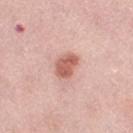<case>
<biopsy_status>not biopsied; imaged during a skin examination</biopsy_status>
<lighting>white-light</lighting>
<patient>
  <sex>female</sex>
  <age_approx>65</age_approx>
</patient>
<image>
  <source>total-body photography crop</source>
  <field_of_view_mm>15</field_of_view_mm>
</image>
<automated_metrics>
  <cielab_L>61</cielab_L>
  <cielab_a>26</cielab_a>
  <cielab_b>28</cielab_b>
  <vs_skin_contrast_norm>8.5</vs_skin_contrast_norm>
  <border_irregularity_0_10>2.0</border_irregularity_0_10>
  <peripheral_color_asymmetry>1.0</peripheral_color_asymmetry>
  <nevus_likeness_0_100>95</nevus_likeness_0_100>
</automated_metrics>
<lesion_size>
  <long_diameter_mm_approx>3.5</long_diameter_mm_approx>
</lesion_size>
<site>leg</site>
</case>